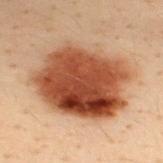Notes:
• workup — no biopsy performed (imaged during a skin exam)
• automated lesion analysis — a border-irregularity index near 2/10 and radial color variation of about 3; a classifier nevus-likeness of about 100/100 and a lesion-detection confidence of about 100/100
• patient — male, approximately 30 years of age
• illumination — cross-polarized
• location — the upper back
• image source — 15 mm crop, total-body photography
• size — about 9.5 mm